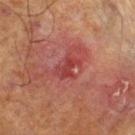Q: Was a biopsy performed?
A: no biopsy performed (imaged during a skin exam)
Q: What is the imaging modality?
A: ~15 mm crop, total-body skin-cancer survey
Q: Where on the body is the lesion?
A: the right lower leg
Q: Who is the patient?
A: male, aged around 70
Q: Illumination type?
A: cross-polarized illumination
Q: What did automated image analysis measure?
A: a lesion color around L≈34 a*≈29 b*≈22 in CIELAB, roughly 7 lightness units darker than nearby skin, and a lesion-to-skin contrast of about 6.5 (normalized; higher = more distinct); border irregularity of about 5.5 on a 0–10 scale and radial color variation of about 1.5